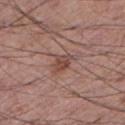The lesion was tiled from a total-body skin photograph and was not biopsied. Automated image analysis of the tile measured a border-irregularity rating of about 3/10 and internal color variation of about 2.5 on a 0–10 scale. And it measured a nevus-likeness score of about 25/100 and lesion-presence confidence of about 100/100. The lesion is on the left lower leg. A male patient, approximately 60 years of age. The recorded lesion diameter is about 2.5 mm. This is a white-light tile. A lesion tile, about 15 mm wide, cut from a 3D total-body photograph.